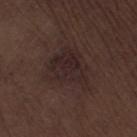Q: Was a biopsy performed?
A: no biopsy performed (imaged during a skin exam)
Q: How was the tile lit?
A: white-light
Q: How large is the lesion?
A: ≈5.5 mm
Q: How was this image acquired?
A: 15 mm crop, total-body photography
Q: Where on the body is the lesion?
A: the right thigh
Q: Patient demographics?
A: male, aged 68–72